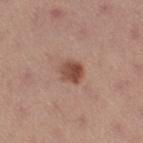Part of a total-body skin-imaging series; this lesion was reviewed on a skin check and was not flagged for biopsy. The patient is a female about 25 years old. Approximately 2.5 mm at its widest. The lesion is on the right thigh. Cropped from a whole-body photographic skin survey; the tile spans about 15 mm. Automated image analysis of the tile measured a shape eccentricity near 0.35 and a symmetry-axis asymmetry near 0.15. The analysis additionally found a classifier nevus-likeness of about 95/100.